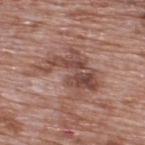Clinical impression: The lesion was tiled from a total-body skin photograph and was not biopsied. Image and clinical context: The lesion-visualizer software estimated a border-irregularity index near 9/10 and a color-variation rating of about 7/10. The software also gave a nevus-likeness score of about 0/100 and lesion-presence confidence of about 80/100. This image is a 15 mm lesion crop taken from a total-body photograph. About 7.5 mm across. A male patient aged 68–72. Captured under white-light illumination. The lesion is on the back.